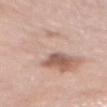No biopsy was performed on this lesion — it was imaged during a full skin examination and was not determined to be concerning. Automated tile analysis of the lesion measured an area of roughly 38 mm², an outline eccentricity of about 0.9 (0 = round, 1 = elongated), and a shape-asymmetry score of about 0.4 (0 = symmetric). The software also gave an average lesion color of about L≈66 a*≈17 b*≈26 (CIELAB), a lesion–skin lightness drop of about 7, and a normalized border contrast of about 4.5. The analysis additionally found a border-irregularity rating of about 8/10, internal color variation of about 10 on a 0–10 scale, and peripheral color asymmetry of about 4.5. A 15 mm crop from a total-body photograph taken for skin-cancer surveillance. About 11.5 mm across. The lesion is on the abdomen. A female subject roughly 65 years of age.